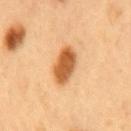Q: Was a biopsy performed?
A: imaged on a skin check; not biopsied
Q: Patient demographics?
A: male, aged 48–52
Q: What is the lesion's diameter?
A: ~4.5 mm (longest diameter)
Q: What is the imaging modality?
A: ~15 mm crop, total-body skin-cancer survey
Q: Automated lesion metrics?
A: a border-irregularity rating of about 1.5/10; a classifier nevus-likeness of about 100/100 and lesion-presence confidence of about 100/100
Q: What is the anatomic site?
A: the mid back